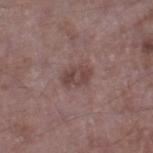The lesion was photographed on a routine skin check and not biopsied; there is no pathology result.